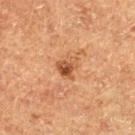No biopsy was performed on this lesion — it was imaged during a full skin examination and was not determined to be concerning.
On the left thigh.
The tile uses cross-polarized illumination.
An algorithmic analysis of the crop reported a mean CIELAB color near L≈42 a*≈21 b*≈32, a lesion–skin lightness drop of about 9, and a normalized lesion–skin contrast near 7.5.
A male subject in their mid- to late 70s.
A 15 mm close-up tile from a total-body photography series done for melanoma screening.
The lesion's longest dimension is about 3 mm.